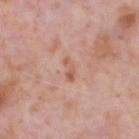Impression: This lesion was catalogued during total-body skin photography and was not selected for biopsy. Acquisition and patient details: The total-body-photography lesion software estimated an eccentricity of roughly 0.8 and a symmetry-axis asymmetry near 0.3. And it measured a border-irregularity index near 3/10, a color-variation rating of about 1.5/10, and peripheral color asymmetry of about 0.5. This is a white-light tile. A male subject, in their 70s. From the head or neck. Cropped from a whole-body photographic skin survey; the tile spans about 15 mm. Longest diameter approximately 2.5 mm.